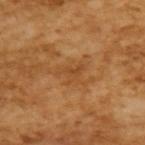No biopsy was performed on this lesion — it was imaged during a full skin examination and was not determined to be concerning.
A male subject approximately 65 years of age.
This is a cross-polarized tile.
A 15 mm close-up extracted from a 3D total-body photography capture.
Automated tile analysis of the lesion measured a lesion area of about 3.5 mm², an outline eccentricity of about 0.8 (0 = round, 1 = elongated), and a shape-asymmetry score of about 0.6 (0 = symmetric). The analysis additionally found a classifier nevus-likeness of about 0/100 and a lesion-detection confidence of about 100/100.